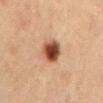Impression: No biopsy was performed on this lesion — it was imaged during a full skin examination and was not determined to be concerning. Image and clinical context: A lesion tile, about 15 mm wide, cut from a 3D total-body photograph. A male patient, aged approximately 65. The lesion's longest dimension is about 3.5 mm. From the mid back. This is a cross-polarized tile.